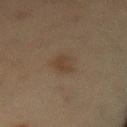An algorithmic analysis of the crop reported an area of roughly 5.5 mm², an outline eccentricity of about 0.8 (0 = round, 1 = elongated), and a symmetry-axis asymmetry near 0.25. A male subject in their mid- to late 60s. Imaged with cross-polarized lighting. A 15 mm crop from a total-body photograph taken for skin-cancer surveillance. The lesion is located on the lower back.